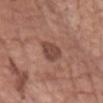This lesion was catalogued during total-body skin photography and was not selected for biopsy.
On the chest.
The tile uses white-light illumination.
The patient is a female about 80 years old.
A roughly 15 mm field-of-view crop from a total-body skin photograph.
About 3 mm across.